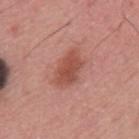Background: Automated image analysis of the tile measured an area of roughly 9.5 mm² and a shape eccentricity near 0.8. And it measured internal color variation of about 2.5 on a 0–10 scale and a peripheral color-asymmetry measure near 1. The software also gave an automated nevus-likeness rating near 90 out of 100 and a detector confidence of about 100 out of 100 that the crop contains a lesion. Captured under white-light illumination. Cropped from a total-body skin-imaging series; the visible field is about 15 mm. The recorded lesion diameter is about 4.5 mm. A male patient aged 53 to 57.